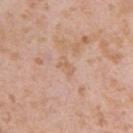Clinical impression:
This lesion was catalogued during total-body skin photography and was not selected for biopsy.
Background:
A lesion tile, about 15 mm wide, cut from a 3D total-body photograph. The tile uses white-light illumination. The lesion's longest dimension is about 2.5 mm. On the left upper arm. A male subject aged approximately 40. The total-body-photography lesion software estimated a footprint of about 3 mm², an eccentricity of roughly 0.85, and a symmetry-axis asymmetry near 0.4. And it measured a border-irregularity index near 3.5/10, internal color variation of about 1 on a 0–10 scale, and peripheral color asymmetry of about 0. It also reported an automated nevus-likeness rating near 0 out of 100.